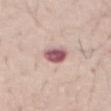Assessment: The lesion was photographed on a routine skin check and not biopsied; there is no pathology result. Context: The lesion is on the chest. The total-body-photography lesion software estimated a lesion color around L≈55 a*≈26 b*≈18 in CIELAB, a lesion–skin lightness drop of about 18, and a normalized lesion–skin contrast near 12. It also reported a nevus-likeness score of about 0/100 and a detector confidence of about 100 out of 100 that the crop contains a lesion. Captured under white-light illumination. This image is a 15 mm lesion crop taken from a total-body photograph. A male patient about 60 years old. About 3 mm across.